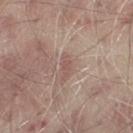workup: catalogued during a skin exam; not biopsied | lighting: white-light | lesion diameter: ~2.5 mm (longest diameter) | image-analysis metrics: an area of roughly 2.5 mm², an eccentricity of roughly 0.9, and a shape-asymmetry score of about 0.25 (0 = symmetric); a lesion color around L≈53 a*≈19 b*≈23 in CIELAB and about 7 CIELAB-L* units darker than the surrounding skin | site: the left thigh | acquisition: ~15 mm crop, total-body skin-cancer survey | subject: male, approximately 65 years of age.